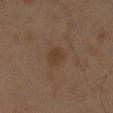biopsy_status: not biopsied; imaged during a skin examination
site: left forearm
image:
  source: total-body photography crop
  field_of_view_mm: 15
patient:
  sex: male
  age_approx: 45